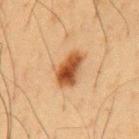<record>
  <biopsy_status>not biopsied; imaged during a skin examination</biopsy_status>
  <lighting>cross-polarized</lighting>
  <lesion_size>
    <long_diameter_mm_approx>4.5</long_diameter_mm_approx>
  </lesion_size>
  <image>
    <source>total-body photography crop</source>
    <field_of_view_mm>15</field_of_view_mm>
  </image>
  <site>chest</site>
  <patient>
    <sex>male</sex>
    <age_approx>60</age_approx>
  </patient>
</record>